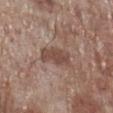Notes:
- workup: total-body-photography surveillance lesion; no biopsy
- automated metrics: a lesion area of about 8 mm² and a shape eccentricity near 0.85; about 10 CIELAB-L* units darker than the surrounding skin and a normalized border contrast of about 7.5; border irregularity of about 2.5 on a 0–10 scale, a within-lesion color-variation index near 2.5/10, and radial color variation of about 1
- patient: male, aged 68–72
- lesion size: ~4 mm (longest diameter)
- imaging modality: total-body-photography crop, ~15 mm field of view
- body site: the right lower leg